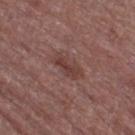Clinical impression:
Captured during whole-body skin photography for melanoma surveillance; the lesion was not biopsied.